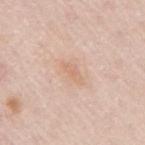Captured during whole-body skin photography for melanoma surveillance; the lesion was not biopsied. Located on the right upper arm. A female patient, aged approximately 65. The recorded lesion diameter is about 3.5 mm. A lesion tile, about 15 mm wide, cut from a 3D total-body photograph. An algorithmic analysis of the crop reported a lesion color around L≈68 a*≈19 b*≈31 in CIELAB, roughly 6 lightness units darker than nearby skin, and a normalized lesion–skin contrast near 5. The software also gave an automated nevus-likeness rating near 0 out of 100 and a detector confidence of about 100 out of 100 that the crop contains a lesion.